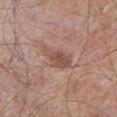Part of a total-body skin-imaging series; this lesion was reviewed on a skin check and was not flagged for biopsy.
An algorithmic analysis of the crop reported an average lesion color of about L≈51 a*≈20 b*≈26 (CIELAB) and a lesion-to-skin contrast of about 6.5 (normalized; higher = more distinct). The software also gave border irregularity of about 3 on a 0–10 scale, a within-lesion color-variation index near 2/10, and a peripheral color-asymmetry measure near 0.5. It also reported an automated nevus-likeness rating near 30 out of 100 and a detector confidence of about 100 out of 100 that the crop contains a lesion.
A male patient roughly 60 years of age.
From the left lower leg.
Approximately 3.5 mm at its widest.
Captured under white-light illumination.
A 15 mm close-up extracted from a 3D total-body photography capture.